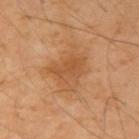Q: Was a biopsy performed?
A: imaged on a skin check; not biopsied
Q: Lesion location?
A: the right upper arm
Q: What are the patient's age and sex?
A: male, aged 48 to 52
Q: How was this image acquired?
A: ~15 mm crop, total-body skin-cancer survey
Q: Illumination type?
A: cross-polarized